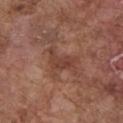Impression:
This lesion was catalogued during total-body skin photography and was not selected for biopsy.
Acquisition and patient details:
This is a white-light tile. A male subject aged 73–77. The lesion-visualizer software estimated roughly 7 lightness units darker than nearby skin and a lesion-to-skin contrast of about 6 (normalized; higher = more distinct). And it measured border irregularity of about 6 on a 0–10 scale and a peripheral color-asymmetry measure near 1. The software also gave a detector confidence of about 100 out of 100 that the crop contains a lesion. Measured at roughly 3.5 mm in maximum diameter. The lesion is located on the chest. A close-up tile cropped from a whole-body skin photograph, about 15 mm across.